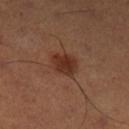workup = no biopsy performed (imaged during a skin exam) | subject = male, in their 50s | anatomic site = the left lower leg | lesion diameter = about 3 mm | image-analysis metrics = a border-irregularity rating of about 2/10, a within-lesion color-variation index near 2/10, and peripheral color asymmetry of about 0.5; a classifier nevus-likeness of about 90/100 and a lesion-detection confidence of about 100/100 | illumination = cross-polarized illumination | image = 15 mm crop, total-body photography.